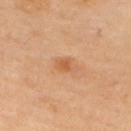Case summary:
• workup: imaged on a skin check; not biopsied
• body site: the upper back
• patient: female, in their 60s
• image-analysis metrics: a shape eccentricity near 0.8; a mean CIELAB color near L≈61 a*≈24 b*≈40, roughly 8 lightness units darker than nearby skin, and a normalized border contrast of about 6; a border-irregularity rating of about 3.5/10, internal color variation of about 1.5 on a 0–10 scale, and a peripheral color-asymmetry measure near 0
• imaging modality: total-body-photography crop, ~15 mm field of view